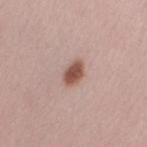<lesion>
<biopsy_status>not biopsied; imaged during a skin examination</biopsy_status>
<site>right upper arm</site>
<image>
  <source>total-body photography crop</source>
  <field_of_view_mm>15</field_of_view_mm>
</image>
<lesion_size>
  <long_diameter_mm_approx>3.0</long_diameter_mm_approx>
</lesion_size>
<patient>
  <sex>female</sex>
  <age_approx>45</age_approx>
</patient>
</lesion>